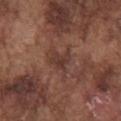{
  "lesion_size": {
    "long_diameter_mm_approx": 2.5
  },
  "site": "chest",
  "patient": {
    "sex": "male",
    "age_approx": 75
  },
  "lighting": "white-light",
  "image": {
    "source": "total-body photography crop",
    "field_of_view_mm": 15
  }
}